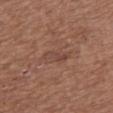workup: total-body-photography surveillance lesion; no biopsy | image: 15 mm crop, total-body photography | diameter: ≈3.5 mm | subject: female, aged 73–77 | lighting: white-light illumination | location: the chest.